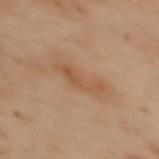Part of a total-body skin-imaging series; this lesion was reviewed on a skin check and was not flagged for biopsy.
Measured at roughly 4.5 mm in maximum diameter.
The patient is a female aged 53 to 57.
On the upper back.
A roughly 15 mm field-of-view crop from a total-body skin photograph.